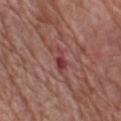Q: Was a biopsy performed?
A: imaged on a skin check; not biopsied
Q: What is the anatomic site?
A: the front of the torso
Q: Automated lesion metrics?
A: a border-irregularity index near 4.5/10, internal color variation of about 2 on a 0–10 scale, and a peripheral color-asymmetry measure near 0.5
Q: What is the lesion's diameter?
A: ~3.5 mm (longest diameter)
Q: Patient demographics?
A: male, aged approximately 65
Q: How was this image acquired?
A: ~15 mm crop, total-body skin-cancer survey